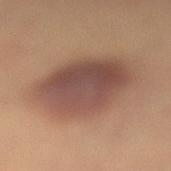- biopsy status: total-body-photography surveillance lesion; no biopsy
- subject: female, aged 33–37
- acquisition: total-body-photography crop, ~15 mm field of view
- location: the leg